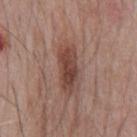{"biopsy_status": "not biopsied; imaged during a skin examination", "patient": {"sex": "male", "age_approx": 70}, "image": {"source": "total-body photography crop", "field_of_view_mm": 15}, "lesion_size": {"long_diameter_mm_approx": 5.0}, "lighting": "white-light", "site": "chest"}